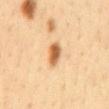Recorded during total-body skin imaging; not selected for excision or biopsy.
Imaged with cross-polarized lighting.
A male patient, in their mid-30s.
A 15 mm close-up tile from a total-body photography series done for melanoma screening.
From the mid back.